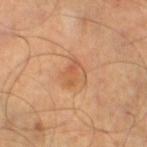Captured during whole-body skin photography for melanoma surveillance; the lesion was not biopsied. The lesion's longest dimension is about 3.5 mm. A male patient, about 45 years old. Automated image analysis of the tile measured a border-irregularity index near 3/10, internal color variation of about 4 on a 0–10 scale, and a peripheral color-asymmetry measure near 1.5. The analysis additionally found lesion-presence confidence of about 100/100. A 15 mm close-up extracted from a 3D total-body photography capture. The lesion is located on the left thigh. The tile uses cross-polarized illumination.